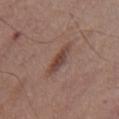notes: no biopsy performed (imaged during a skin exam) | imaging modality: total-body-photography crop, ~15 mm field of view | location: the mid back | subject: male, in their 60s | tile lighting: white-light illumination | size: ~3.5 mm (longest diameter) | TBP lesion metrics: a lesion-to-skin contrast of about 7.5 (normalized; higher = more distinct); border irregularity of about 2.5 on a 0–10 scale, internal color variation of about 3.5 on a 0–10 scale, and a peripheral color-asymmetry measure near 1; a classifier nevus-likeness of about 80/100 and a lesion-detection confidence of about 100/100.